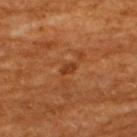| field | value |
|---|---|
| workup | catalogued during a skin exam; not biopsied |
| image | ~15 mm tile from a whole-body skin photo |
| anatomic site | the upper back |
| size | ≈2 mm |
| subject | male, approximately 60 years of age |
| lighting | cross-polarized illumination |